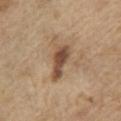Notes:
* biopsy status — catalogued during a skin exam; not biopsied
* imaging modality — 15 mm crop, total-body photography
* subject — male, aged 68–72
* anatomic site — the arm